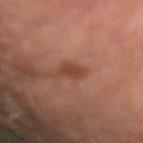Assessment:
This lesion was catalogued during total-body skin photography and was not selected for biopsy.